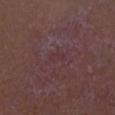Case summary:
* tile lighting · white-light illumination
* patient · male, about 60 years old
* lesion size · ~2 mm (longest diameter)
* imaging modality · ~15 mm crop, total-body skin-cancer survey
* image-analysis metrics · an eccentricity of roughly 0.75 and two-axis asymmetry of about 0.3; border irregularity of about 3 on a 0–10 scale and peripheral color asymmetry of about 0.5
* location · the head or neck
* histopathology · a squamous cell carcinoma in situ